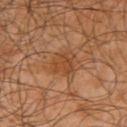Imaged during a routine full-body skin examination; the lesion was not biopsied and no histopathology is available. A roughly 15 mm field-of-view crop from a total-body skin photograph. A male subject aged approximately 65. Located on the right upper arm.